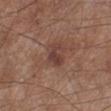{"biopsy_status": "not biopsied; imaged during a skin examination", "site": "left lower leg", "automated_metrics": {"cielab_L": 40, "cielab_a": 20, "cielab_b": 23, "vs_skin_contrast_norm": 7.5, "border_irregularity_0_10": 5.0, "nevus_likeness_0_100": 5, "lesion_detection_confidence_0_100": 100}, "patient": {"sex": "male", "age_approx": 55}, "image": {"source": "total-body photography crop", "field_of_view_mm": 15}}